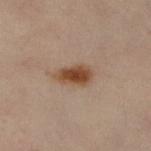{
  "biopsy_status": "not biopsied; imaged during a skin examination",
  "site": "left leg",
  "patient": {
    "sex": "female",
    "age_approx": 60
  },
  "image": {
    "source": "total-body photography crop",
    "field_of_view_mm": 15
  },
  "lighting": "cross-polarized"
}